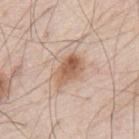Captured during whole-body skin photography for melanoma surveillance; the lesion was not biopsied. Located on the mid back. A male subject roughly 80 years of age. The recorded lesion diameter is about 4 mm. The lesion-visualizer software estimated a lesion color around L≈59 a*≈19 b*≈31 in CIELAB, roughly 12 lightness units darker than nearby skin, and a normalized lesion–skin contrast near 8.5. The software also gave border irregularity of about 2.5 on a 0–10 scale, a color-variation rating of about 6/10, and radial color variation of about 2. Captured under white-light illumination. A region of skin cropped from a whole-body photographic capture, roughly 15 mm wide.